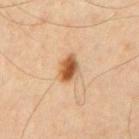Q: What is the imaging modality?
A: total-body-photography crop, ~15 mm field of view
Q: What did automated image analysis measure?
A: an area of roughly 6 mm² and a shape-asymmetry score of about 0.2 (0 = symmetric); a border-irregularity rating of about 2/10 and peripheral color asymmetry of about 2
Q: Lesion location?
A: the chest
Q: Patient demographics?
A: male, in their mid- to late 40s
Q: Lesion size?
A: ~3.5 mm (longest diameter)
Q: How was the tile lit?
A: cross-polarized illumination